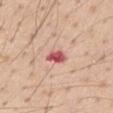{"biopsy_status": "not biopsied; imaged during a skin examination", "image": {"source": "total-body photography crop", "field_of_view_mm": 15}, "site": "right upper arm", "lighting": "white-light", "patient": {"sex": "male", "age_approx": 55}, "automated_metrics": {"vs_skin_darker_L": 17.0, "vs_skin_contrast_norm": 10.5, "border_irregularity_0_10": 2.0, "color_variation_0_10": 3.5, "peripheral_color_asymmetry": 1.5}}